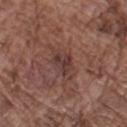Acquisition and patient details:
About 4.5 mm across. This is a white-light tile. A male patient in their mid- to late 70s. A roughly 15 mm field-of-view crop from a total-body skin photograph. Located on the left forearm.